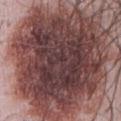The lesion was photographed on a routine skin check and not biopsied; there is no pathology result.
Captured under white-light illumination.
Longest diameter approximately 14 mm.
This image is a 15 mm lesion crop taken from a total-body photograph.
A female subject aged approximately 50.
Automated tile analysis of the lesion measured a footprint of about 120 mm² and a shape eccentricity near 0.55. And it measured a border-irregularity rating of about 3.5/10. The analysis additionally found an automated nevus-likeness rating near 0 out of 100 and a lesion-detection confidence of about 100/100.
On the abdomen.